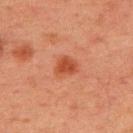<tbp_lesion>
  <biopsy_status>not biopsied; imaged during a skin examination</biopsy_status>
  <image>
    <source>total-body photography crop</source>
    <field_of_view_mm>15</field_of_view_mm>
  </image>
  <patient>
    <sex>male</sex>
    <age_approx>60</age_approx>
  </patient>
  <site>upper back</site>
  <automated_metrics>
    <border_irregularity_0_10>2.5</border_irregularity_0_10>
    <color_variation_0_10>2.0</color_variation_0_10>
    <peripheral_color_asymmetry>0.5</peripheral_color_asymmetry>
    <nevus_likeness_0_100>95</nevus_likeness_0_100>
  </automated_metrics>
  <lesion_size>
    <long_diameter_mm_approx>2.5</long_diameter_mm_approx>
  </lesion_size>
  <lighting>cross-polarized</lighting>
</tbp_lesion>